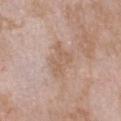{
  "biopsy_status": "not biopsied; imaged during a skin examination",
  "lighting": "white-light",
  "site": "chest",
  "automated_metrics": {
    "vs_skin_darker_L": 7.0,
    "vs_skin_contrast_norm": 5.5
  },
  "patient": {
    "sex": "male",
    "age_approx": 50
  },
  "image": {
    "source": "total-body photography crop",
    "field_of_view_mm": 15
  },
  "lesion_size": {
    "long_diameter_mm_approx": 4.5
  }
}